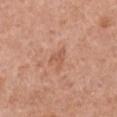Clinical impression: Part of a total-body skin-imaging series; this lesion was reviewed on a skin check and was not flagged for biopsy. Background: A female subject in their mid- to late 60s. A 15 mm close-up tile from a total-body photography series done for melanoma screening. The lesion is located on the chest. About 2.5 mm across.